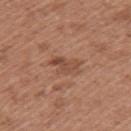notes — no biopsy performed (imaged during a skin exam); image — 15 mm crop, total-body photography; site — the left upper arm; patient — female, aged approximately 50.